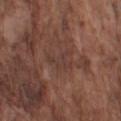{
  "biopsy_status": "not biopsied; imaged during a skin examination",
  "site": "left upper arm",
  "patient": {
    "sex": "male",
    "age_approx": 75
  },
  "lesion_size": {
    "long_diameter_mm_approx": 3.5
  },
  "image": {
    "source": "total-body photography crop",
    "field_of_view_mm": 15
  },
  "lighting": "white-light"
}